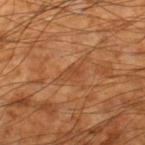{"biopsy_status": "not biopsied; imaged during a skin examination", "patient": {"sex": "male", "age_approx": 60}, "lesion_size": {"long_diameter_mm_approx": 3.0}, "automated_metrics": {"color_variation_0_10": 2.0, "peripheral_color_asymmetry": 1.0}, "image": {"source": "total-body photography crop", "field_of_view_mm": 15}, "lighting": "cross-polarized", "site": "right lower leg"}